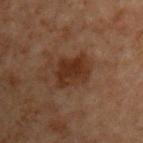Clinical impression: Imaged during a routine full-body skin examination; the lesion was not biopsied and no histopathology is available. Acquisition and patient details: Captured under cross-polarized illumination. On the chest. This image is a 15 mm lesion crop taken from a total-body photograph. The subject is a male roughly 70 years of age. The lesion's longest dimension is about 4 mm.